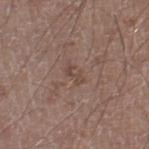notes = total-body-photography surveillance lesion; no biopsy | image = 15 mm crop, total-body photography | automated metrics = an automated nevus-likeness rating near 0 out of 100 and a lesion-detection confidence of about 100/100 | patient = male, about 20 years old | tile lighting = white-light | body site = the right lower leg.